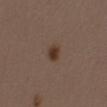  automated_metrics:
    border_irregularity_0_10: 2.0
    color_variation_0_10: 2.5
    peripheral_color_asymmetry: 1.0
  lighting: white-light
  image:
    source: total-body photography crop
    field_of_view_mm: 15
  patient:
    sex: male
    age_approx: 40
  site: front of the torso